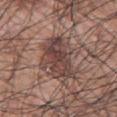Findings:
• follow-up — imaged on a skin check; not biopsied
• diameter — ≈6.5 mm
• tile lighting — white-light
• subject — male, approximately 70 years of age
• image source — ~15 mm tile from a whole-body skin photo
• anatomic site — the chest
• automated lesion analysis — a lesion area of about 21 mm², a shape eccentricity near 0.75, and a symmetry-axis asymmetry near 0.25; a lesion color around L≈43 a*≈18 b*≈21 in CIELAB, a lesion–skin lightness drop of about 11, and a normalized lesion–skin contrast near 8.5; a border-irregularity index near 3/10, a color-variation rating of about 6/10, and radial color variation of about 2; a nevus-likeness score of about 5/100